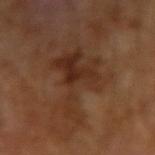biopsy_status: not biopsied; imaged during a skin examination
patient:
  sex: male
  age_approx: 65
image:
  source: total-body photography crop
  field_of_view_mm: 15
lighting: cross-polarized
automated_metrics:
  cielab_L: 30
  cielab_a: 19
  cielab_b: 27
  vs_skin_darker_L: 7.0
  vs_skin_contrast_norm: 7.0
  lesion_detection_confidence_0_100: 100
lesion_size:
  long_diameter_mm_approx: 8.5